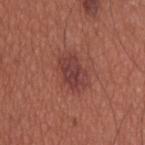Imaged during a routine full-body skin examination; the lesion was not biopsied and no histopathology is available. The lesion is on the upper back. A male patient aged approximately 35. An algorithmic analysis of the crop reported a symmetry-axis asymmetry near 0.2. The software also gave border irregularity of about 3 on a 0–10 scale and a within-lesion color-variation index near 3/10. This is a white-light tile. The lesion's longest dimension is about 4 mm. A 15 mm close-up extracted from a 3D total-body photography capture.